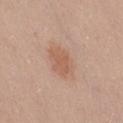Part of a total-body skin-imaging series; this lesion was reviewed on a skin check and was not flagged for biopsy.
Automated tile analysis of the lesion measured border irregularity of about 2.5 on a 0–10 scale and internal color variation of about 2 on a 0–10 scale.
The tile uses white-light illumination.
A roughly 15 mm field-of-view crop from a total-body skin photograph.
The lesion is on the right thigh.
A female subject, approximately 30 years of age.
About 3.5 mm across.